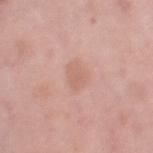biopsy status: no biopsy performed (imaged during a skin exam)
lesion diameter: ≈3 mm
body site: the right thigh
lighting: white-light illumination
image-analysis metrics: an area of roughly 5 mm², an outline eccentricity of about 0.65 (0 = round, 1 = elongated), and a symmetry-axis asymmetry near 0.3; a lesion color around L≈63 a*≈22 b*≈27 in CIELAB and about 6 CIELAB-L* units darker than the surrounding skin; border irregularity of about 2.5 on a 0–10 scale and radial color variation of about 0.5; an automated nevus-likeness rating near 0 out of 100
subject: female, aged around 50
image source: total-body-photography crop, ~15 mm field of view